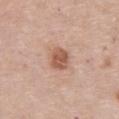Captured during whole-body skin photography for melanoma surveillance; the lesion was not biopsied. From the front of the torso. A 15 mm close-up tile from a total-body photography series done for melanoma screening. Measured at roughly 3 mm in maximum diameter. The subject is a female in their 60s.